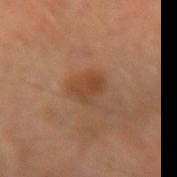Q: How was this image acquired?
A: 15 mm crop, total-body photography
Q: Lesion location?
A: the right forearm
Q: Patient demographics?
A: male, roughly 40 years of age